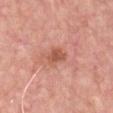Recorded during total-body skin imaging; not selected for excision or biopsy. A male subject, aged around 45. The lesion-visualizer software estimated an eccentricity of roughly 0.7 and two-axis asymmetry of about 0.4. The software also gave a lesion–skin lightness drop of about 10 and a lesion-to-skin contrast of about 7.5 (normalized; higher = more distinct). Cropped from a total-body skin-imaging series; the visible field is about 15 mm. About 2.5 mm across. Located on the front of the torso. Captured under white-light illumination.